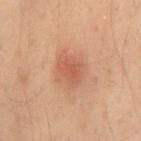Clinical impression:
This lesion was catalogued during total-body skin photography and was not selected for biopsy.
Image and clinical context:
This is a cross-polarized tile. This image is a 15 mm lesion crop taken from a total-body photograph. About 3 mm across. On the mid back. An algorithmic analysis of the crop reported a lesion area of about 5 mm², a shape eccentricity near 0.7, and a shape-asymmetry score of about 0.3 (0 = symmetric). The patient is a male roughly 40 years of age.